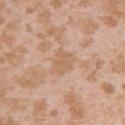Imaged during a routine full-body skin examination; the lesion was not biopsied and no histopathology is available. Automated tile analysis of the lesion measured a lesion area of about 5.5 mm² and a shape eccentricity near 0.65. It also reported a border-irregularity index near 2.5/10 and radial color variation of about 0.5. The tile uses white-light illumination. From the left upper arm. A region of skin cropped from a whole-body photographic capture, roughly 15 mm wide. The patient is a female in their mid- to late 20s.